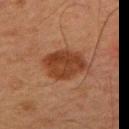Q: Was this lesion biopsied?
A: catalogued during a skin exam; not biopsied
Q: What is the anatomic site?
A: the back
Q: What kind of image is this?
A: 15 mm crop, total-body photography
Q: Illumination type?
A: cross-polarized
Q: What are the patient's age and sex?
A: male, in their mid-60s